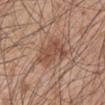{
  "biopsy_status": "not biopsied; imaged during a skin examination",
  "patient": {
    "sex": "male",
    "age_approx": 45
  },
  "lighting": "white-light",
  "image": {
    "source": "total-body photography crop",
    "field_of_view_mm": 15
  },
  "site": "left upper arm",
  "lesion_size": {
    "long_diameter_mm_approx": 5.5
  }
}